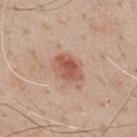Case summary:
• image source — ~15 mm tile from a whole-body skin photo
• subject — male, aged around 55
• site — the chest